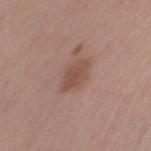workup = no biopsy performed (imaged during a skin exam) | image = ~15 mm tile from a whole-body skin photo | location = the chest | diameter = about 3.5 mm | TBP lesion metrics = a footprint of about 6.5 mm² and a symmetry-axis asymmetry near 0.2; a mean CIELAB color near L≈49 a*≈20 b*≈25, a lesion–skin lightness drop of about 9, and a normalized lesion–skin contrast near 6.5 | tile lighting = white-light | subject = male, approximately 30 years of age.